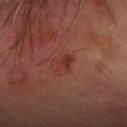Q: Is there a histopathology result?
A: no biopsy performed (imaged during a skin exam)
Q: Patient demographics?
A: male, aged 68 to 72
Q: How was this image acquired?
A: 15 mm crop, total-body photography
Q: What is the anatomic site?
A: the left forearm
Q: What lighting was used for the tile?
A: cross-polarized illumination
Q: How large is the lesion?
A: about 3 mm